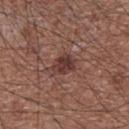{
  "biopsy_status": "not biopsied; imaged during a skin examination",
  "patient": {
    "sex": "male",
    "age_approx": 75
  },
  "site": "chest",
  "lighting": "white-light",
  "lesion_size": {
    "long_diameter_mm_approx": 3.0
  },
  "automated_metrics": {
    "lesion_detection_confidence_0_100": 100
  },
  "image": {
    "source": "total-body photography crop",
    "field_of_view_mm": 15
  }
}